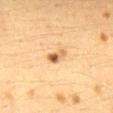Case summary:
- notes · total-body-photography surveillance lesion; no biopsy
- subject · female, roughly 40 years of age
- image source · ~15 mm tile from a whole-body skin photo
- anatomic site · the back